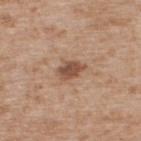workup: imaged on a skin check; not biopsied | automated metrics: an average lesion color of about L≈51 a*≈20 b*≈30 (CIELAB), a lesion–skin lightness drop of about 12, and a normalized lesion–skin contrast near 8.5; an automated nevus-likeness rating near 75 out of 100 and lesion-presence confidence of about 100/100 | lesion diameter: ~3 mm (longest diameter) | image source: total-body-photography crop, ~15 mm field of view | subject: male, aged 63 to 67 | tile lighting: white-light illumination | body site: the upper back.